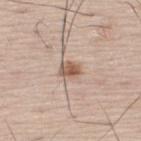image source = total-body-photography crop, ~15 mm field of view | anatomic site = the back | image-analysis metrics = peripheral color asymmetry of about 1.5 | size = ≈2.5 mm | subject = male, aged approximately 75 | illumination = white-light.